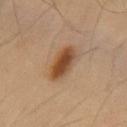biopsy_status: not biopsied; imaged during a skin examination
lesion_size:
  long_diameter_mm_approx: 4.5
image:
  source: total-body photography crop
  field_of_view_mm: 15
site: mid back
patient:
  sex: male
  age_approx: 55
lighting: cross-polarized